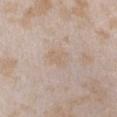No biopsy was performed on this lesion — it was imaged during a full skin examination and was not determined to be concerning. Captured under white-light illumination. Measured at roughly 3 mm in maximum diameter. The lesion is on the chest. A female subject, aged around 25. A 15 mm close-up extracted from a 3D total-body photography capture.